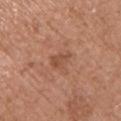| field | value |
|---|---|
| follow-up | total-body-photography surveillance lesion; no biopsy |
| anatomic site | the chest |
| image source | total-body-photography crop, ~15 mm field of view |
| lesion size | ≈2.5 mm |
| subject | male, about 75 years old |